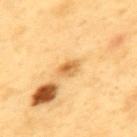The lesion was photographed on a routine skin check and not biopsied; there is no pathology result.
The total-body-photography lesion software estimated a nevus-likeness score of about 0/100 and lesion-presence confidence of about 100/100.
The lesion is on the upper back.
This is a cross-polarized tile.
Measured at roughly 2.5 mm in maximum diameter.
A 15 mm close-up extracted from a 3D total-body photography capture.
The subject is a male aged approximately 60.